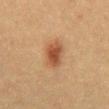Imaged during a routine full-body skin examination; the lesion was not biopsied and no histopathology is available.
The patient is a male aged approximately 50.
Located on the abdomen.
This is a cross-polarized tile.
A 15 mm close-up tile from a total-body photography series done for melanoma screening.